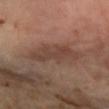{"biopsy_status": "not biopsied; imaged during a skin examination", "site": "right forearm", "lighting": "cross-polarized", "image": {"source": "total-body photography crop", "field_of_view_mm": 15}, "patient": {"sex": "female", "age_approx": 45}, "lesion_size": {"long_diameter_mm_approx": 6.5}}